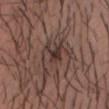Q: Is there a histopathology result?
A: total-body-photography surveillance lesion; no biopsy
Q: What are the patient's age and sex?
A: male, about 30 years old
Q: What is the anatomic site?
A: the chest
Q: What is the imaging modality?
A: ~15 mm crop, total-body skin-cancer survey